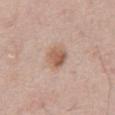No biopsy was performed on this lesion — it was imaged during a full skin examination and was not determined to be concerning. The subject is a male aged 63 to 67. Approximately 3 mm at its widest. This image is a 15 mm lesion crop taken from a total-body photograph. Automated tile analysis of the lesion measured an average lesion color of about L≈58 a*≈19 b*≈29 (CIELAB), a lesion–skin lightness drop of about 10, and a normalized border contrast of about 7.5. And it measured a nevus-likeness score of about 90/100 and a detector confidence of about 100 out of 100 that the crop contains a lesion. Located on the abdomen.